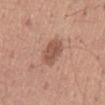Part of a total-body skin-imaging series; this lesion was reviewed on a skin check and was not flagged for biopsy.
This image is a 15 mm lesion crop taken from a total-body photograph.
A male subject, in their mid-50s.
The lesion-visualizer software estimated a lesion area of about 7.5 mm², an outline eccentricity of about 0.85 (0 = round, 1 = elongated), and two-axis asymmetry of about 0.1. It also reported an average lesion color of about L≈53 a*≈22 b*≈28 (CIELAB), roughly 11 lightness units darker than nearby skin, and a normalized border contrast of about 7.5. The analysis additionally found a border-irregularity rating of about 1.5/10. And it measured lesion-presence confidence of about 100/100.
The lesion's longest dimension is about 4 mm.
Located on the mid back.
The tile uses white-light illumination.